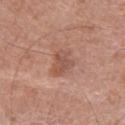This lesion was catalogued during total-body skin photography and was not selected for biopsy. This image is a 15 mm lesion crop taken from a total-body photograph. This is a white-light tile. The lesion is on the right lower leg. About 3.5 mm across. A male subject aged 58 to 62.